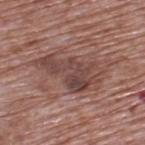Clinical impression: Captured during whole-body skin photography for melanoma surveillance; the lesion was not biopsied. Image and clinical context: A 15 mm close-up extracted from a 3D total-body photography capture. This is a white-light tile. The lesion is on the upper back. About 7.5 mm across. A male patient, about 70 years old.